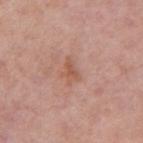Clinical impression: This lesion was catalogued during total-body skin photography and was not selected for biopsy. Clinical summary: Imaged with white-light lighting. The lesion is located on the right upper arm. A roughly 15 mm field-of-view crop from a total-body skin photograph. A female patient aged approximately 60.